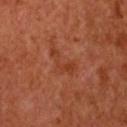biopsy status=catalogued during a skin exam; not biopsied
illumination=cross-polarized
lesion diameter=about 4 mm
subject=female, aged around 65
anatomic site=the chest
image=~15 mm crop, total-body skin-cancer survey